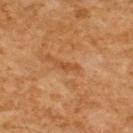Findings:
* follow-up · imaged on a skin check; not biopsied
* TBP lesion metrics · a shape eccentricity near 0.95 and a shape-asymmetry score of about 0.35 (0 = symmetric); a border-irregularity index near 4/10, a within-lesion color-variation index near 0/10, and peripheral color asymmetry of about 0; a classifier nevus-likeness of about 0/100 and a detector confidence of about 95 out of 100 that the crop contains a lesion
* lesion size · ~3 mm (longest diameter)
* imaging modality · ~15 mm crop, total-body skin-cancer survey
* illumination · cross-polarized
* subject · male, roughly 60 years of age
* site · the upper back